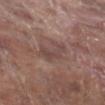Impression:
This lesion was catalogued during total-body skin photography and was not selected for biopsy.
Acquisition and patient details:
Cropped from a whole-body photographic skin survey; the tile spans about 15 mm. A male subject, about 70 years old. The lesion is located on the right lower leg.